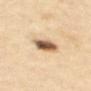Assessment: Captured during whole-body skin photography for melanoma surveillance; the lesion was not biopsied. Image and clinical context: A roughly 15 mm field-of-view crop from a total-body skin photograph. The lesion is located on the upper back. Measured at roughly 3.5 mm in maximum diameter. Imaged with cross-polarized lighting. The subject is a female aged 58–62.